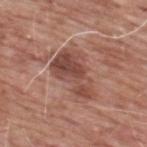  biopsy_status: not biopsied; imaged during a skin examination
  image:
    source: total-body photography crop
    field_of_view_mm: 15
  site: back
  lesion_size:
    long_diameter_mm_approx: 6.0
  automated_metrics:
    border_irregularity_0_10: 4.5
    color_variation_0_10: 6.0
    peripheral_color_asymmetry: 2.0
    nevus_likeness_0_100: 10
    lesion_detection_confidence_0_100: 100
  lighting: white-light
  patient:
    sex: male
    age_approx: 60